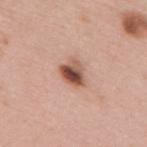Imaged during a routine full-body skin examination; the lesion was not biopsied and no histopathology is available. The recorded lesion diameter is about 3.5 mm. A 15 mm crop from a total-body photograph taken for skin-cancer surveillance. Captured under white-light illumination. The lesion is located on the upper back. A female patient, in their 40s.